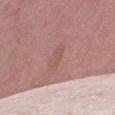notes: imaged on a skin check; not biopsied | diameter: ~3 mm (longest diameter) | patient: female, aged 38 to 42 | illumination: white-light illumination | image source: 15 mm crop, total-body photography | location: the right thigh | image-analysis metrics: an average lesion color of about L≈54 a*≈22 b*≈25 (CIELAB) and a lesion-to-skin contrast of about 4.5 (normalized; higher = more distinct); a border-irregularity rating of about 4/10, a within-lesion color-variation index near 0/10, and peripheral color asymmetry of about 0; an automated nevus-likeness rating near 0 out of 100 and lesion-presence confidence of about 95/100.